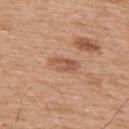Clinical impression: The lesion was tiled from a total-body skin photograph and was not biopsied. Background: Captured under white-light illumination. A 15 mm close-up extracted from a 3D total-body photography capture. The recorded lesion diameter is about 3.5 mm. On the back. A male patient aged 63–67. Automated image analysis of the tile measured a border-irregularity index near 2.5/10, a within-lesion color-variation index near 3.5/10, and a peripheral color-asymmetry measure near 1.